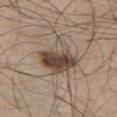Clinical impression:
Captured during whole-body skin photography for melanoma surveillance; the lesion was not biopsied.
Image and clinical context:
On the left thigh. A male patient aged 58–62. Automated tile analysis of the lesion measured a border-irregularity rating of about 3/10. It also reported an automated nevus-likeness rating near 95 out of 100 and a detector confidence of about 100 out of 100 that the crop contains a lesion. A 15 mm close-up extracted from a 3D total-body photography capture.